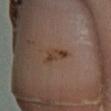Case summary:
* follow-up — imaged on a skin check; not biopsied
* acquisition — ~15 mm crop, total-body skin-cancer survey
* size — ~4 mm (longest diameter)
* tile lighting — cross-polarized
* image-analysis metrics — an eccentricity of roughly 0.9 and a shape-asymmetry score of about 0.4 (0 = symmetric); internal color variation of about 3 on a 0–10 scale and a peripheral color-asymmetry measure near 0.5; lesion-presence confidence of about 100/100
* subject — male, in their 40s
* location — the left lower leg